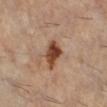The lesion was tiled from a total-body skin photograph and was not biopsied.
A female patient, aged around 60.
Cropped from a whole-body photographic skin survey; the tile spans about 15 mm.
The lesion is on the right lower leg.